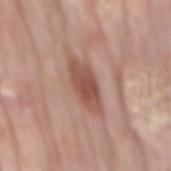workup = catalogued during a skin exam; not biopsied | location = the mid back | size = ≈5.5 mm | subject = male, in their mid-70s | lighting = white-light illumination | image-analysis metrics = a mean CIELAB color near L≈52 a*≈22 b*≈27, about 12 CIELAB-L* units darker than the surrounding skin, and a normalized border contrast of about 8 | acquisition = ~15 mm crop, total-body skin-cancer survey.